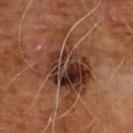Assessment: Captured during whole-body skin photography for melanoma surveillance; the lesion was not biopsied. Clinical summary: The tile uses cross-polarized illumination. Cropped from a whole-body photographic skin survey; the tile spans about 15 mm. The recorded lesion diameter is about 8.5 mm. From the front of the torso. A male subject aged 58–62.